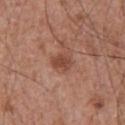* follow-up · imaged on a skin check; not biopsied
* image-analysis metrics · a lesion area of about 4.5 mm², a shape eccentricity near 0.6, and two-axis asymmetry of about 0.25
* lesion size · about 2.5 mm
* acquisition · total-body-photography crop, ~15 mm field of view
* lighting · white-light illumination
* body site · the chest
* patient · male, aged 63–67